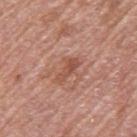follow-up: total-body-photography surveillance lesion; no biopsy | site: the left upper arm | image source: 15 mm crop, total-body photography | subject: female, approximately 60 years of age | automated lesion analysis: a mean CIELAB color near L≈51 a*≈24 b*≈29 and a lesion-to-skin contrast of about 6 (normalized; higher = more distinct); a color-variation rating of about 2/10 and radial color variation of about 0.5; an automated nevus-likeness rating near 0 out of 100 and lesion-presence confidence of about 100/100 | lesion diameter: about 3.5 mm.